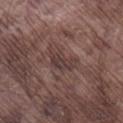Clinical impression: The lesion was photographed on a routine skin check and not biopsied; there is no pathology result.